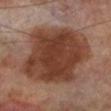  biopsy_status: not biopsied; imaged during a skin examination
  site: left lower leg
  lighting: cross-polarized
  image:
    source: total-body photography crop
    field_of_view_mm: 15
  automated_metrics:
    area_mm2_approx: 60.0
    eccentricity: 0.6
    shape_asymmetry: 0.2
    vs_skin_darker_L: 13.0
    vs_skin_contrast_norm: 11.0
  lesion_size:
    long_diameter_mm_approx: 9.0
  patient:
    sex: male
    age_approx: 70